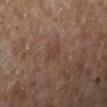Captured during whole-body skin photography for melanoma surveillance; the lesion was not biopsied. A 15 mm close-up tile from a total-body photography series done for melanoma screening. Imaged with cross-polarized lighting. The total-body-photography lesion software estimated a border-irregularity rating of about 2/10, internal color variation of about 0.5 on a 0–10 scale, and radial color variation of about 0. And it measured a lesion-detection confidence of about 100/100. The lesion's longest dimension is about 2.5 mm. The subject is a female in their 60s. The lesion is located on the right lower leg.